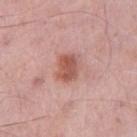Assessment:
The lesion was tiled from a total-body skin photograph and was not biopsied.
Image and clinical context:
About 3 mm across. Automated tile analysis of the lesion measured a mean CIELAB color near L≈55 a*≈25 b*≈27 and a normalized lesion–skin contrast near 8. The analysis additionally found a border-irregularity index near 2/10, a within-lesion color-variation index near 3.5/10, and radial color variation of about 1. The software also gave a classifier nevus-likeness of about 90/100 and a detector confidence of about 100 out of 100 that the crop contains a lesion. From the left thigh. Imaged with white-light lighting. A close-up tile cropped from a whole-body skin photograph, about 15 mm across. A male subject aged around 55.